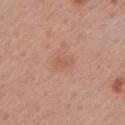biopsy status=no biopsy performed (imaged during a skin exam) | automated metrics=an area of roughly 3 mm², an eccentricity of roughly 0.8, and a symmetry-axis asymmetry near 0.35; border irregularity of about 3.5 on a 0–10 scale, internal color variation of about 2 on a 0–10 scale, and peripheral color asymmetry of about 1; an automated nevus-likeness rating near 0 out of 100 | image=~15 mm tile from a whole-body skin photo | subject=female, approximately 55 years of age | lighting=white-light | size=about 2.5 mm | body site=the left upper arm.